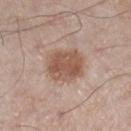Q: Is there a histopathology result?
A: imaged on a skin check; not biopsied
Q: Patient demographics?
A: male, approximately 60 years of age
Q: What lighting was used for the tile?
A: white-light
Q: Lesion location?
A: the right lower leg
Q: How was this image acquired?
A: 15 mm crop, total-body photography